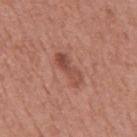– biopsy status · imaged on a skin check; not biopsied
– patient · male, in their mid-50s
– lesion size · about 4.5 mm
– image-analysis metrics · an area of roughly 5.5 mm², an outline eccentricity of about 0.95 (0 = round, 1 = elongated), and a symmetry-axis asymmetry near 0.3; a mean CIELAB color near L≈49 a*≈25 b*≈28 and a lesion-to-skin contrast of about 7 (normalized; higher = more distinct); border irregularity of about 4 on a 0–10 scale and a within-lesion color-variation index near 5.5/10; an automated nevus-likeness rating near 20 out of 100 and lesion-presence confidence of about 100/100
– anatomic site · the mid back
– acquisition · ~15 mm tile from a whole-body skin photo